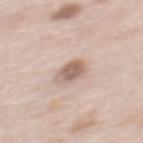Q: Was a biopsy performed?
A: imaged on a skin check; not biopsied
Q: What did automated image analysis measure?
A: a footprint of about 5.5 mm²; roughly 13 lightness units darker than nearby skin and a normalized border contrast of about 8
Q: What is the imaging modality?
A: 15 mm crop, total-body photography
Q: What is the anatomic site?
A: the upper back
Q: What is the lesion's diameter?
A: ~3 mm (longest diameter)
Q: What lighting was used for the tile?
A: white-light illumination
Q: Who is the patient?
A: female, aged approximately 65